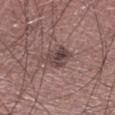biopsy status: catalogued during a skin exam; not biopsied
anatomic site: the right lower leg
lesion diameter: ~3 mm (longest diameter)
tile lighting: white-light illumination
subject: male, aged around 60
acquisition: ~15 mm crop, total-body skin-cancer survey
automated lesion analysis: an outline eccentricity of about 0.5 (0 = round, 1 = elongated) and two-axis asymmetry of about 0.2; border irregularity of about 2 on a 0–10 scale and radial color variation of about 2; an automated nevus-likeness rating near 65 out of 100 and lesion-presence confidence of about 100/100